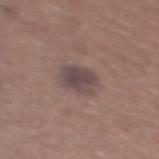<record>
<biopsy_status>not biopsied; imaged during a skin examination</biopsy_status>
<lesion_size>
  <long_diameter_mm_approx>3.5</long_diameter_mm_approx>
</lesion_size>
<patient>
  <sex>female</sex>
  <age_approx>65</age_approx>
</patient>
<lighting>white-light</lighting>
<site>upper back</site>
<image>
  <source>total-body photography crop</source>
  <field_of_view_mm>15</field_of_view_mm>
</image>
</record>